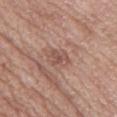Q: Was this lesion biopsied?
A: total-body-photography surveillance lesion; no biopsy